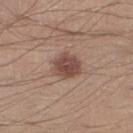Captured during whole-body skin photography for melanoma surveillance; the lesion was not biopsied. The lesion is located on the right lower leg. A 15 mm close-up tile from a total-body photography series done for melanoma screening. A male subject, roughly 30 years of age.